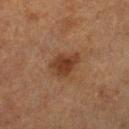Findings:
- notes · total-body-photography surveillance lesion; no biopsy
- lesion size · about 4 mm
- image source · 15 mm crop, total-body photography
- site · the leg
- subject · male, in their 70s
- automated lesion analysis · a footprint of about 8 mm², an eccentricity of roughly 0.75, and a symmetry-axis asymmetry near 0.25; a border-irregularity rating of about 2.5/10, a color-variation rating of about 2.5/10, and radial color variation of about 0.5; a classifier nevus-likeness of about 90/100 and a lesion-detection confidence of about 100/100
- lighting · cross-polarized illumination